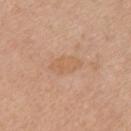follow-up=catalogued during a skin exam; not biopsied | anatomic site=the chest | patient=female, in their mid- to late 50s | diameter=~4 mm (longest diameter) | acquisition=~15 mm tile from a whole-body skin photo | lighting=white-light illumination.